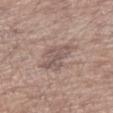workup: imaged on a skin check; not biopsied | lesion diameter: ≈3.5 mm | body site: the left forearm | automated lesion analysis: a lesion color around L≈54 a*≈15 b*≈21 in CIELAB and roughly 8 lightness units darker than nearby skin; a nevus-likeness score of about 0/100 and a lesion-detection confidence of about 100/100 | lighting: white-light illumination | subject: male, roughly 55 years of age | image: ~15 mm tile from a whole-body skin photo.